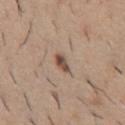<case>
<biopsy_status>not biopsied; imaged during a skin examination</biopsy_status>
<patient>
  <sex>male</sex>
  <age_approx>40</age_approx>
</patient>
<lesion_size>
  <long_diameter_mm_approx>2.5</long_diameter_mm_approx>
</lesion_size>
<lighting>white-light</lighting>
<automated_metrics>
  <border_irregularity_0_10>2.0</border_irregularity_0_10>
  <color_variation_0_10>6.0</color_variation_0_10>
  <peripheral_color_asymmetry>1.5</peripheral_color_asymmetry>
</automated_metrics>
<image>
  <source>total-body photography crop</source>
  <field_of_view_mm>15</field_of_view_mm>
</image>
<site>front of the torso</site>
</case>